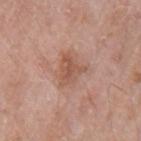Assessment:
This lesion was catalogued during total-body skin photography and was not selected for biopsy.
Acquisition and patient details:
About 3.5 mm across. A region of skin cropped from a whole-body photographic capture, roughly 15 mm wide. The lesion is on the left upper arm. A male patient aged 63 to 67. The tile uses white-light illumination.